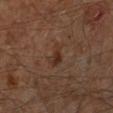This image is a 15 mm lesion crop taken from a total-body photograph. The recorded lesion diameter is about 2.5 mm. Imaged with cross-polarized lighting. The lesion is on the left lower leg. A male subject, aged approximately 60.